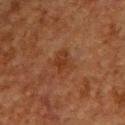Part of a total-body skin-imaging series; this lesion was reviewed on a skin check and was not flagged for biopsy. A roughly 15 mm field-of-view crop from a total-body skin photograph. Located on the back. This is a cross-polarized tile. A male subject, aged 48 to 52.